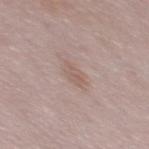Notes:
- follow-up: catalogued during a skin exam; not biopsied
- anatomic site: the back
- tile lighting: white-light illumination
- diameter: about 3 mm
- patient: male, aged 48 to 52
- automated lesion analysis: a lesion–skin lightness drop of about 6; a border-irregularity index near 2.5/10, internal color variation of about 2 on a 0–10 scale, and radial color variation of about 0.5
- image source: 15 mm crop, total-body photography